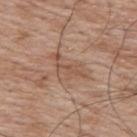A region of skin cropped from a whole-body photographic capture, roughly 15 mm wide.
The lesion is on the upper back.
A male subject, aged 68–72.